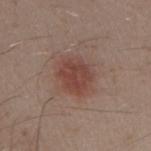Assessment: The lesion was tiled from a total-body skin photograph and was not biopsied. Context: A male subject, approximately 30 years of age. From the right upper arm. A 15 mm close-up extracted from a 3D total-body photography capture.